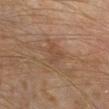Part of a total-body skin-imaging series; this lesion was reviewed on a skin check and was not flagged for biopsy.
A male subject aged 58–62.
Automated tile analysis of the lesion measured an eccentricity of roughly 0.45 and a symmetry-axis asymmetry near 0.45. And it measured a lesion–skin lightness drop of about 5 and a normalized border contrast of about 4.5. It also reported internal color variation of about 1.5 on a 0–10 scale and radial color variation of about 0.5.
Cropped from a whole-body photographic skin survey; the tile spans about 15 mm.
Located on the leg.